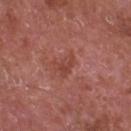{
  "biopsy_status": "not biopsied; imaged during a skin examination",
  "patient": {
    "sex": "male",
    "age_approx": 65
  },
  "image": {
    "source": "total-body photography crop",
    "field_of_view_mm": 15
  },
  "site": "chest"
}